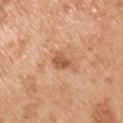| key | value |
|---|---|
| workup | no biopsy performed (imaged during a skin exam) |
| body site | the right upper arm |
| diameter | ~2.5 mm (longest diameter) |
| imaging modality | total-body-photography crop, ~15 mm field of view |
| patient | male, aged approximately 55 |